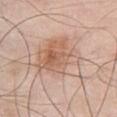Clinical impression:
This lesion was catalogued during total-body skin photography and was not selected for biopsy.
Background:
The lesion is on the front of the torso. The patient is a male in their mid-50s. The lesion's longest dimension is about 7 mm. Captured under white-light illumination. The lesion-visualizer software estimated an area of roughly 24 mm², an eccentricity of roughly 0.75, and a symmetry-axis asymmetry near 0.3. The analysis additionally found an average lesion color of about L≈62 a*≈19 b*≈29 (CIELAB) and about 8 CIELAB-L* units darker than the surrounding skin. A 15 mm crop from a total-body photograph taken for skin-cancer surveillance.